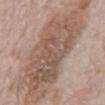This lesion was catalogued during total-body skin photography and was not selected for biopsy. A male subject roughly 70 years of age. From the abdomen. Automated tile analysis of the lesion measured a mean CIELAB color near L≈55 a*≈16 b*≈26. The analysis additionally found a border-irregularity rating of about 5.5/10, a within-lesion color-variation index near 4.5/10, and radial color variation of about 1.5. It also reported an automated nevus-likeness rating near 0 out of 100 and lesion-presence confidence of about 95/100. About 16.5 mm across. A roughly 15 mm field-of-view crop from a total-body skin photograph.